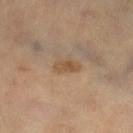Part of a total-body skin-imaging series; this lesion was reviewed on a skin check and was not flagged for biopsy. A 15 mm close-up extracted from a 3D total-body photography capture. Automated image analysis of the tile measured a lesion area of about 4 mm², a shape eccentricity near 0.85, and two-axis asymmetry of about 0.25. The software also gave a lesion color around L≈51 a*≈17 b*≈33 in CIELAB and a lesion–skin lightness drop of about 8. It also reported a nevus-likeness score of about 55/100 and a detector confidence of about 100 out of 100 that the crop contains a lesion. The lesion is on the right lower leg. The patient is approximately 60 years of age.